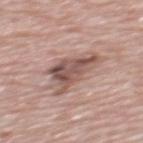biopsy status: total-body-photography surveillance lesion; no biopsy
imaging modality: ~15 mm crop, total-body skin-cancer survey
location: the mid back
patient: male, roughly 70 years of age
illumination: white-light